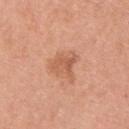Imaged during a routine full-body skin examination; the lesion was not biopsied and no histopathology is available. This is a white-light tile. A male subject aged 28–32. From the back. A close-up tile cropped from a whole-body skin photograph, about 15 mm across. An algorithmic analysis of the crop reported a mean CIELAB color near L≈58 a*≈26 b*≈34 and a lesion–skin lightness drop of about 9. The analysis additionally found border irregularity of about 4 on a 0–10 scale and peripheral color asymmetry of about 0.5. The analysis additionally found a detector confidence of about 100 out of 100 that the crop contains a lesion. Approximately 3 mm at its widest.